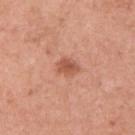Assessment: Recorded during total-body skin imaging; not selected for excision or biopsy. Clinical summary: Measured at roughly 3 mm in maximum diameter. The total-body-photography lesion software estimated a shape eccentricity near 0.7 and a symmetry-axis asymmetry near 0.25. It also reported an average lesion color of about L≈55 a*≈27 b*≈32 (CIELAB), roughly 10 lightness units darker than nearby skin, and a lesion-to-skin contrast of about 7 (normalized; higher = more distinct). It also reported a border-irregularity index near 2.5/10, internal color variation of about 2 on a 0–10 scale, and peripheral color asymmetry of about 0.5. The software also gave a nevus-likeness score of about 85/100 and a lesion-detection confidence of about 100/100. The lesion is located on the upper back. The patient is a male aged 48–52. A lesion tile, about 15 mm wide, cut from a 3D total-body photograph.